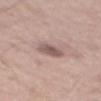notes: no biopsy performed (imaged during a skin exam); location: the mid back; patient: male, about 55 years old; acquisition: total-body-photography crop, ~15 mm field of view; automated lesion analysis: a border-irregularity rating of about 2.5/10, a within-lesion color-variation index near 3.5/10, and a peripheral color-asymmetry measure near 1.